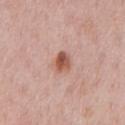biopsy_status: not biopsied; imaged during a skin examination
site: chest
automated_metrics:
  area_mm2_approx: 5.0
  eccentricity: 0.65
  cielab_L: 55
  cielab_a: 24
  cielab_b: 29
  vs_skin_darker_L: 13.0
  vs_skin_contrast_norm: 9.0
  nevus_likeness_0_100: 95
  lesion_detection_confidence_0_100: 100
image:
  source: total-body photography crop
  field_of_view_mm: 15
lesion_size:
  long_diameter_mm_approx: 3.0
patient:
  sex: male
  age_approx: 50